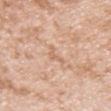Clinical impression: Part of a total-body skin-imaging series; this lesion was reviewed on a skin check and was not flagged for biopsy. Clinical summary: The lesion is located on the arm. Imaged with white-light lighting. A roughly 15 mm field-of-view crop from a total-body skin photograph. A male subject aged 38 to 42. Approximately 2.5 mm at its widest.